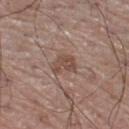  site: left lower leg
  automated_metrics:
    area_mm2_approx: 5.5
    eccentricity: 0.6
    shape_asymmetry: 0.3
    border_irregularity_0_10: 3.0
    color_variation_0_10: 3.0
  lesion_size:
    long_diameter_mm_approx: 3.0
  patient:
    sex: male
    age_approx: 70
  image:
    source: total-body photography crop
    field_of_view_mm: 15